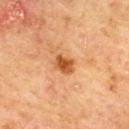Part of a total-body skin-imaging series; this lesion was reviewed on a skin check and was not flagged for biopsy. Automated image analysis of the tile measured an area of roughly 4.5 mm², an eccentricity of roughly 0.65, and a symmetry-axis asymmetry near 0.15. It also reported a lesion color around L≈45 a*≈23 b*≈37 in CIELAB, about 11 CIELAB-L* units darker than the surrounding skin, and a normalized lesion–skin contrast near 9. It also reported border irregularity of about 1.5 on a 0–10 scale and a color-variation rating of about 2.5/10. A roughly 15 mm field-of-view crop from a total-body skin photograph. A male patient, aged 68–72. On the mid back. Imaged with cross-polarized lighting.